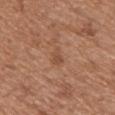Case summary:
- notes · no biopsy performed (imaged during a skin exam)
- body site · the upper back
- imaging modality · ~15 mm tile from a whole-body skin photo
- patient · male, approximately 70 years of age
- lighting · white-light
- lesion size · about 3 mm
- TBP lesion metrics · a lesion color around L≈50 a*≈22 b*≈31 in CIELAB, roughly 7 lightness units darker than nearby skin, and a normalized border contrast of about 5; a classifier nevus-likeness of about 0/100 and lesion-presence confidence of about 100/100